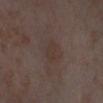Clinical impression: Part of a total-body skin-imaging series; this lesion was reviewed on a skin check and was not flagged for biopsy. Clinical summary: A close-up tile cropped from a whole-body skin photograph, about 15 mm across. On the left lower leg. This is a cross-polarized tile. The patient is a female aged 53–57. Approximately 2.5 mm at its widest.